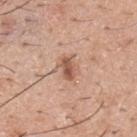Assessment:
This lesion was catalogued during total-body skin photography and was not selected for biopsy.
Image and clinical context:
The patient is a male in their 40s. About 3 mm across. On the upper back. Captured under white-light illumination. Cropped from a whole-body photographic skin survey; the tile spans about 15 mm.